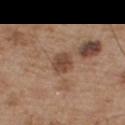Clinical impression: Imaged during a routine full-body skin examination; the lesion was not biopsied and no histopathology is available. Context: A male subject approximately 55 years of age. From the front of the torso. Cropped from a whole-body photographic skin survey; the tile spans about 15 mm.